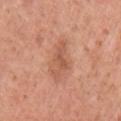Assessment:
This lesion was catalogued during total-body skin photography and was not selected for biopsy.
Context:
A 15 mm crop from a total-body photograph taken for skin-cancer surveillance. Captured under white-light illumination. A female patient aged around 35. The lesion is located on the left upper arm. About 4 mm across.